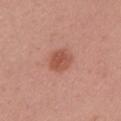The lesion was photographed on a routine skin check and not biopsied; there is no pathology result. On the left upper arm. A close-up tile cropped from a whole-body skin photograph, about 15 mm across. A female subject aged 33 to 37.